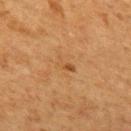Assessment: No biopsy was performed on this lesion — it was imaged during a full skin examination and was not determined to be concerning. Image and clinical context: A female subject about 50 years old. Approximately 2.5 mm at its widest. Automated tile analysis of the lesion measured a mean CIELAB color near L≈45 a*≈19 b*≈36, roughly 5 lightness units darker than nearby skin, and a normalized lesion–skin contrast near 5. The software also gave border irregularity of about 4 on a 0–10 scale, internal color variation of about 1 on a 0–10 scale, and a peripheral color-asymmetry measure near 0. From the upper back. Captured under cross-polarized illumination. A 15 mm close-up tile from a total-body photography series done for melanoma screening.